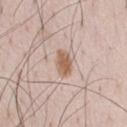<case>
<biopsy_status>not biopsied; imaged during a skin examination</biopsy_status>
<lesion_size>
  <long_diameter_mm_approx>3.0</long_diameter_mm_approx>
</lesion_size>
<lighting>white-light</lighting>
<site>chest</site>
<patient>
  <sex>male</sex>
  <age_approx>35</age_approx>
</patient>
<image>
  <source>total-body photography crop</source>
  <field_of_view_mm>15</field_of_view_mm>
</image>
</case>